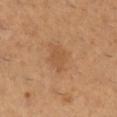{"biopsy_status": "not biopsied; imaged during a skin examination", "patient": {"sex": "female", "age_approx": 55}, "image": {"source": "total-body photography crop", "field_of_view_mm": 15}, "lighting": "cross-polarized", "automated_metrics": {"cielab_L": 53, "cielab_a": 21, "cielab_b": 37, "vs_skin_darker_L": 6.0, "vs_skin_contrast_norm": 5.0, "nevus_likeness_0_100": 0}, "site": "leg"}